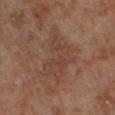Notes:
• subject: male, aged approximately 70
• diameter: ≈6.5 mm
• TBP lesion metrics: an eccentricity of roughly 0.8 and two-axis asymmetry of about 0.45
• tile lighting: cross-polarized
• anatomic site: the left lower leg
• image: total-body-photography crop, ~15 mm field of view